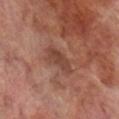A region of skin cropped from a whole-body photographic capture, roughly 15 mm wide. From the leg. A male subject, aged approximately 70.